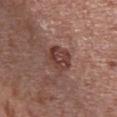Captured during whole-body skin photography for melanoma surveillance; the lesion was not biopsied. The tile uses white-light illumination. From the chest. This image is a 15 mm lesion crop taken from a total-body photograph. The lesion's longest dimension is about 3.5 mm. A male patient aged 63–67.